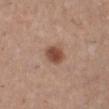Notes:
• notes · imaged on a skin check; not biopsied
• lighting · white-light
• image · ~15 mm tile from a whole-body skin photo
• diameter · ~3 mm (longest diameter)
• patient · female, aged 28 to 32
• site · the right lower leg
• automated metrics · a lesion color around L≈48 a*≈21 b*≈28 in CIELAB and a lesion-to-skin contrast of about 9.5 (normalized; higher = more distinct); an automated nevus-likeness rating near 100 out of 100